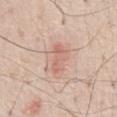The lesion was tiled from a total-body skin photograph and was not biopsied.